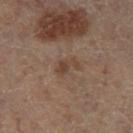- workup · catalogued during a skin exam; not biopsied
- lesion diameter · ~3 mm (longest diameter)
- imaging modality · total-body-photography crop, ~15 mm field of view
- site · the leg
- image-analysis metrics · about 7 CIELAB-L* units darker than the surrounding skin
- patient · female, aged 58–62
- lighting · cross-polarized